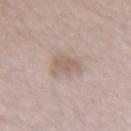This lesion was catalogued during total-body skin photography and was not selected for biopsy.
A close-up tile cropped from a whole-body skin photograph, about 15 mm across.
The recorded lesion diameter is about 3 mm.
Imaged with white-light lighting.
The lesion is on the arm.
The total-body-photography lesion software estimated a footprint of about 5.5 mm² and an eccentricity of roughly 0.45. The analysis additionally found about 8 CIELAB-L* units darker than the surrounding skin and a lesion-to-skin contrast of about 5.5 (normalized; higher = more distinct).
A female patient, in their mid- to late 30s.